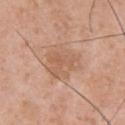Assessment:
No biopsy was performed on this lesion — it was imaged during a full skin examination and was not determined to be concerning.
Context:
A male patient, in their mid- to late 50s. A lesion tile, about 15 mm wide, cut from a 3D total-body photograph. On the back.